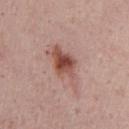notes: no biopsy performed (imaged during a skin exam) | anatomic site: the front of the torso | subject: male, aged 73–77 | acquisition: ~15 mm tile from a whole-body skin photo.